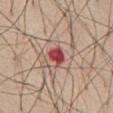Captured during whole-body skin photography for melanoma surveillance; the lesion was not biopsied. The lesion-visualizer software estimated a shape eccentricity near 0.5 and two-axis asymmetry of about 0.25. It also reported an average lesion color of about L≈48 a*≈34 b*≈25 (CIELAB), a lesion–skin lightness drop of about 15, and a normalized border contrast of about 10.5. The analysis additionally found a color-variation rating of about 3.5/10 and radial color variation of about 1. A male patient, aged 58 to 62. This is a white-light tile. A 15 mm crop from a total-body photograph taken for skin-cancer surveillance. On the abdomen. About 2.5 mm across.